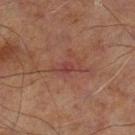Findings:
• notes — catalogued during a skin exam; not biopsied
• imaging modality — 15 mm crop, total-body photography
• automated lesion analysis — a lesion color around L≈33 a*≈21 b*≈20 in CIELAB, roughly 5 lightness units darker than nearby skin, and a lesion-to-skin contrast of about 6 (normalized; higher = more distinct); a color-variation rating of about 2.5/10 and radial color variation of about 1; an automated nevus-likeness rating near 0 out of 100 and lesion-presence confidence of about 100/100
• subject — male, aged around 70
• anatomic site — the left lower leg
• diameter — ~3.5 mm (longest diameter)